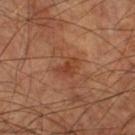biopsy status: no biopsy performed (imaged during a skin exam) | subject: male, about 60 years old | site: the leg | lesion size: ~3.5 mm (longest diameter) | imaging modality: ~15 mm crop, total-body skin-cancer survey | tile lighting: cross-polarized illumination.